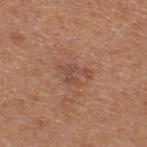Part of a total-body skin-imaging series; this lesion was reviewed on a skin check and was not flagged for biopsy. The total-body-photography lesion software estimated a lesion area of about 5 mm² and an outline eccentricity of about 0.8 (0 = round, 1 = elongated). The analysis additionally found a lesion–skin lightness drop of about 7 and a normalized lesion–skin contrast near 5.5. The software also gave border irregularity of about 6.5 on a 0–10 scale and a peripheral color-asymmetry measure near 1. A male subject aged 63–67. The tile uses white-light illumination. A roughly 15 mm field-of-view crop from a total-body skin photograph. Approximately 3.5 mm at its widest. Located on the upper back.